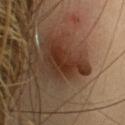Impression:
Captured during whole-body skin photography for melanoma surveillance; the lesion was not biopsied.
Background:
On the head or neck. Captured under cross-polarized illumination. A female subject aged 38 to 42. Cropped from a whole-body photographic skin survey; the tile spans about 15 mm. Measured at roughly 5.5 mm in maximum diameter.